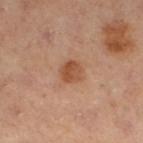Part of a total-body skin-imaging series; this lesion was reviewed on a skin check and was not flagged for biopsy. The tile uses cross-polarized illumination. A female patient, aged 58 to 62. The lesion is on the right leg. A 15 mm crop from a total-body photograph taken for skin-cancer surveillance. Automated tile analysis of the lesion measured a mean CIELAB color near L≈43 a*≈20 b*≈29, a lesion–skin lightness drop of about 9, and a lesion-to-skin contrast of about 7.5 (normalized; higher = more distinct). Approximately 3 mm at its widest.